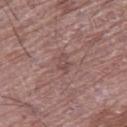Imaged during a routine full-body skin examination; the lesion was not biopsied and no histopathology is available. A male subject aged approximately 75. Longest diameter approximately 2.5 mm. Imaged with white-light lighting. On the right thigh. A close-up tile cropped from a whole-body skin photograph, about 15 mm across.